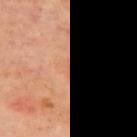Q: Was this lesion biopsied?
A: imaged on a skin check; not biopsied
Q: What kind of image is this?
A: ~15 mm tile from a whole-body skin photo
Q: What is the anatomic site?
A: the upper back
Q: What lighting was used for the tile?
A: cross-polarized
Q: Lesion size?
A: ≈2 mm
Q: Patient demographics?
A: female, about 50 years old